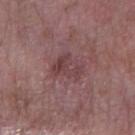| field | value |
|---|---|
| patient | male, approximately 60 years of age |
| TBP lesion metrics | an average lesion color of about L≈41 a*≈21 b*≈17 (CIELAB), roughly 7 lightness units darker than nearby skin, and a normalized lesion–skin contrast near 6; border irregularity of about 4 on a 0–10 scale, a within-lesion color-variation index near 4.5/10, and peripheral color asymmetry of about 1.5; an automated nevus-likeness rating near 0 out of 100 and lesion-presence confidence of about 100/100 |
| anatomic site | the arm |
| imaging modality | 15 mm crop, total-body photography |